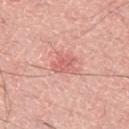Q: Is there a histopathology result?
A: catalogued during a skin exam; not biopsied
Q: Automated lesion metrics?
A: a lesion color around L≈63 a*≈30 b*≈27 in CIELAB and about 9 CIELAB-L* units darker than the surrounding skin; an automated nevus-likeness rating near 0 out of 100 and a lesion-detection confidence of about 100/100
Q: What are the patient's age and sex?
A: male, aged around 50
Q: What is the anatomic site?
A: the left thigh
Q: How was the tile lit?
A: white-light illumination
Q: How large is the lesion?
A: about 3 mm
Q: What kind of image is this?
A: total-body-photography crop, ~15 mm field of view